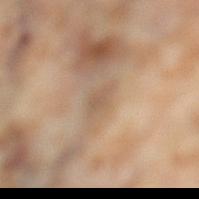Imaged during a routine full-body skin examination; the lesion was not biopsied and no histopathology is available.
The lesion is located on the left lower leg.
This image is a 15 mm lesion crop taken from a total-body photograph.
Captured under cross-polarized illumination.
Measured at roughly 3 mm in maximum diameter.
A female patient approximately 55 years of age.